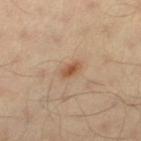No biopsy was performed on this lesion — it was imaged during a full skin examination and was not determined to be concerning. On the left thigh. The recorded lesion diameter is about 2.5 mm. Cropped from a total-body skin-imaging series; the visible field is about 15 mm. The tile uses cross-polarized illumination. A male subject, in their 40s.